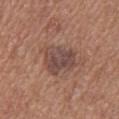Q: Was a biopsy performed?
A: imaged on a skin check; not biopsied
Q: Patient demographics?
A: female, in their mid- to late 50s
Q: How large is the lesion?
A: about 4.5 mm
Q: How was this image acquired?
A: ~15 mm tile from a whole-body skin photo
Q: Lesion location?
A: the upper back
Q: Illumination type?
A: white-light